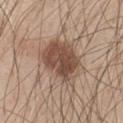No biopsy was performed on this lesion — it was imaged during a full skin examination and was not determined to be concerning. A male subject, aged approximately 45. On the front of the torso. About 6 mm across. This is a white-light tile. A lesion tile, about 15 mm wide, cut from a 3D total-body photograph.